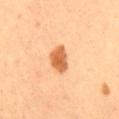Q: Was this lesion biopsied?
A: catalogued during a skin exam; not biopsied
Q: What are the patient's age and sex?
A: female, aged 48–52
Q: What lighting was used for the tile?
A: cross-polarized illumination
Q: How was this image acquired?
A: ~15 mm tile from a whole-body skin photo
Q: Automated lesion metrics?
A: an outline eccentricity of about 0.75 (0 = round, 1 = elongated); a lesion color around L≈65 a*≈28 b*≈45 in CIELAB and a normalized border contrast of about 9.5; internal color variation of about 3 on a 0–10 scale and a peripheral color-asymmetry measure near 1